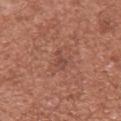Q: Is there a histopathology result?
A: catalogued during a skin exam; not biopsied
Q: Patient demographics?
A: female, roughly 40 years of age
Q: Lesion location?
A: the upper back
Q: What kind of image is this?
A: 15 mm crop, total-body photography
Q: What did automated image analysis measure?
A: a shape eccentricity near 0.85 and two-axis asymmetry of about 0.45; a lesion color around L≈46 a*≈24 b*≈26 in CIELAB, about 7 CIELAB-L* units darker than the surrounding skin, and a lesion-to-skin contrast of about 5.5 (normalized; higher = more distinct); a within-lesion color-variation index near 1/10 and a peripheral color-asymmetry measure near 0.5; a nevus-likeness score of about 0/100 and a detector confidence of about 100 out of 100 that the crop contains a lesion
Q: What is the lesion's diameter?
A: about 3 mm
Q: How was the tile lit?
A: white-light illumination